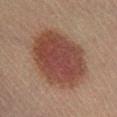A close-up tile cropped from a whole-body skin photograph, about 15 mm across. About 8 mm across. Imaged with cross-polarized lighting. Located on the leg. A male patient, aged around 40. An algorithmic analysis of the crop reported an area of roughly 38 mm², an eccentricity of roughly 0.65, and a shape-asymmetry score of about 0.1 (0 = symmetric). The software also gave a nevus-likeness score of about 100/100 and a detector confidence of about 100 out of 100 that the crop contains a lesion.